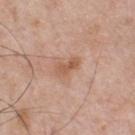* location: the chest
* image source: total-body-photography crop, ~15 mm field of view
* subject: male, in their 50s
* lesion diameter: ≈3 mm